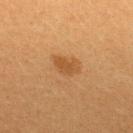Imaged during a routine full-body skin examination; the lesion was not biopsied and no histopathology is available. Cropped from a whole-body photographic skin survey; the tile spans about 15 mm. On the upper back. A female subject aged approximately 20.